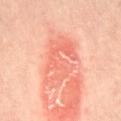Imaged during a routine full-body skin examination; the lesion was not biopsied and no histopathology is available. A region of skin cropped from a whole-body photographic capture, roughly 15 mm wide. A male patient aged 38 to 42. Longest diameter approximately 6 mm. Located on the back. The tile uses cross-polarized illumination. The total-body-photography lesion software estimated two-axis asymmetry of about 0.35. It also reported an average lesion color of about L≈68 a*≈33 b*≈34 (CIELAB), a lesion–skin lightness drop of about 8, and a normalized lesion–skin contrast near 5. And it measured a classifier nevus-likeness of about 5/100 and lesion-presence confidence of about 100/100.